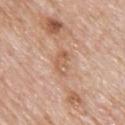Case summary:
* biopsy status · no biopsy performed (imaged during a skin exam)
* lesion size · about 3.5 mm
* site · the upper back
* TBP lesion metrics · a classifier nevus-likeness of about 0/100
* lighting · white-light
* acquisition · total-body-photography crop, ~15 mm field of view
* subject · male, about 75 years old